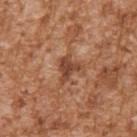Assessment: Part of a total-body skin-imaging series; this lesion was reviewed on a skin check and was not flagged for biopsy. Image and clinical context: From the right upper arm. Cropped from a whole-body photographic skin survey; the tile spans about 15 mm. The recorded lesion diameter is about 3 mm. A male patient about 45 years old.